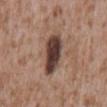Imaged during a routine full-body skin examination; the lesion was not biopsied and no histopathology is available. Approximately 6 mm at its widest. The patient is a male aged approximately 45. This image is a 15 mm lesion crop taken from a total-body photograph. Automated image analysis of the tile measured an area of roughly 13 mm², a shape eccentricity near 0.9, and a symmetry-axis asymmetry near 0.2. And it measured a normalized lesion–skin contrast near 13.5. The software also gave a classifier nevus-likeness of about 70/100 and lesion-presence confidence of about 100/100. The lesion is located on the back.